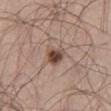Part of a total-body skin-imaging series; this lesion was reviewed on a skin check and was not flagged for biopsy. A 15 mm close-up extracted from a 3D total-body photography capture. A male patient, roughly 25 years of age. The lesion is on the left thigh.